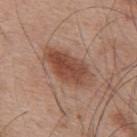Part of a total-body skin-imaging series; this lesion was reviewed on a skin check and was not flagged for biopsy. A 15 mm close-up tile from a total-body photography series done for melanoma screening. On the upper back. A male subject in their mid-50s. The tile uses white-light illumination.